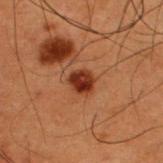Impression: This lesion was catalogued during total-body skin photography and was not selected for biopsy. Image and clinical context: A male patient in their 50s. The tile uses cross-polarized illumination. A close-up tile cropped from a whole-body skin photograph, about 15 mm across. The lesion is on the upper back. The lesion-visualizer software estimated a footprint of about 5.5 mm². It also reported a mean CIELAB color near L≈26 a*≈23 b*≈27, about 12 CIELAB-L* units darker than the surrounding skin, and a normalized border contrast of about 12. The analysis additionally found a border-irregularity rating of about 2/10, a within-lesion color-variation index near 4/10, and radial color variation of about 1.5. The software also gave a classifier nevus-likeness of about 100/100 and a detector confidence of about 100 out of 100 that the crop contains a lesion.